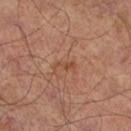Assessment: This lesion was catalogued during total-body skin photography and was not selected for biopsy. Context: A male subject in their mid-60s. This is a cross-polarized tile. A close-up tile cropped from a whole-body skin photograph, about 15 mm across. On the right lower leg. The lesion's longest dimension is about 2.5 mm.